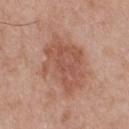Clinical impression:
Part of a total-body skin-imaging series; this lesion was reviewed on a skin check and was not flagged for biopsy.
Clinical summary:
A male subject about 55 years old. A 15 mm close-up extracted from a 3D total-body photography capture. On the upper back. About 6.5 mm across.